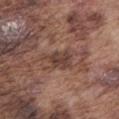Impression: Captured during whole-body skin photography for melanoma surveillance; the lesion was not biopsied. Image and clinical context: The patient is a male aged approximately 75. Located on the back. A 15 mm close-up tile from a total-body photography series done for melanoma screening.